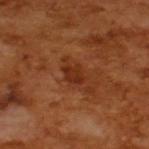Recorded during total-body skin imaging; not selected for excision or biopsy. Approximately 2.5 mm at its widest. The patient is a male aged approximately 65. Imaged with cross-polarized lighting. Automated tile analysis of the lesion measured a footprint of about 4.5 mm², a shape eccentricity near 0.65, and a symmetry-axis asymmetry near 0.25. The analysis additionally found an average lesion color of about L≈30 a*≈26 b*≈33 (CIELAB), about 8 CIELAB-L* units darker than the surrounding skin, and a normalized lesion–skin contrast near 7.5. Cropped from a total-body skin-imaging series; the visible field is about 15 mm.